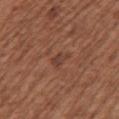biopsy status=no biopsy performed (imaged during a skin exam)
image=15 mm crop, total-body photography
illumination=white-light illumination
body site=the left upper arm
automated metrics=an area of roughly 3.5 mm² and a symmetry-axis asymmetry near 0.3; lesion-presence confidence of about 100/100
size=~2.5 mm (longest diameter)
patient=female, aged 73 to 77